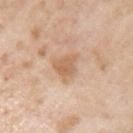Imaged during a routine full-body skin examination; the lesion was not biopsied and no histopathology is available. The recorded lesion diameter is about 3.5 mm. A male patient aged approximately 60. The lesion is on the left upper arm. Cropped from a total-body skin-imaging series; the visible field is about 15 mm. The tile uses white-light illumination.